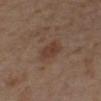{
  "biopsy_status": "not biopsied; imaged during a skin examination",
  "site": "right lower leg",
  "patient": {
    "sex": "female",
    "age_approx": 55
  },
  "lesion_size": {
    "long_diameter_mm_approx": 3.0
  },
  "lighting": "cross-polarized",
  "image": {
    "source": "total-body photography crop",
    "field_of_view_mm": 15
  },
  "automated_metrics": {
    "cielab_L": 38,
    "cielab_a": 18,
    "cielab_b": 26,
    "vs_skin_darker_L": 7.0,
    "vs_skin_contrast_norm": 6.5
  }
}